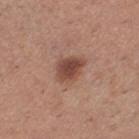Q: Is there a histopathology result?
A: imaged on a skin check; not biopsied
Q: How was the tile lit?
A: white-light illumination
Q: What is the imaging modality?
A: ~15 mm crop, total-body skin-cancer survey
Q: Who is the patient?
A: female, in their 30s
Q: Lesion size?
A: about 3.5 mm
Q: What is the anatomic site?
A: the leg